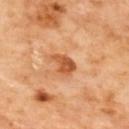<record>
  <biopsy_status>not biopsied; imaged during a skin examination</biopsy_status>
  <site>back</site>
  <image>
    <source>total-body photography crop</source>
    <field_of_view_mm>15</field_of_view_mm>
  </image>
  <automated_metrics>
    <eccentricity>0.7</eccentricity>
    <shape_asymmetry>0.35</shape_asymmetry>
    <border_irregularity_0_10>3.5</border_irregularity_0_10>
    <color_variation_0_10>3.5</color_variation_0_10>
    <peripheral_color_asymmetry>1.5</peripheral_color_asymmetry>
  </automated_metrics>
  <lighting>cross-polarized</lighting>
  <lesion_size>
    <long_diameter_mm_approx>3.5</long_diameter_mm_approx>
  </lesion_size>
</record>